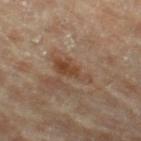<lesion>
<biopsy_status>not biopsied; imaged during a skin examination</biopsy_status>
<image>
  <source>total-body photography crop</source>
  <field_of_view_mm>15</field_of_view_mm>
</image>
<site>leg</site>
<patient>
  <sex>male</sex>
  <age_approx>85+</age_approx>
</patient>
</lesion>